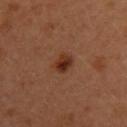Assessment: Captured during whole-body skin photography for melanoma surveillance; the lesion was not biopsied. Image and clinical context: A female subject, about 35 years old. The lesion is on the left upper arm. Longest diameter approximately 2.5 mm. A roughly 15 mm field-of-view crop from a total-body skin photograph. The tile uses cross-polarized illumination.